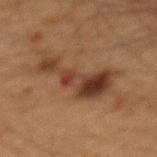Recorded during total-body skin imaging; not selected for excision or biopsy. The subject is a male aged 63–67. This image is a 15 mm lesion crop taken from a total-body photograph. This is a cross-polarized tile. The lesion is on the mid back. The recorded lesion diameter is about 7 mm.